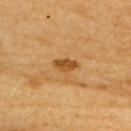follow-up = no biopsy performed (imaged during a skin exam)
patient = female, approximately 55 years of age
imaging modality = ~15 mm tile from a whole-body skin photo
tile lighting = cross-polarized illumination
site = the right upper arm